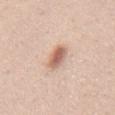workup=imaged on a skin check; not biopsied | size=≈3.5 mm | patient=male, about 35 years old | anatomic site=the abdomen | imaging modality=total-body-photography crop, ~15 mm field of view.